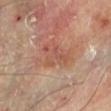Clinical impression:
Part of a total-body skin-imaging series; this lesion was reviewed on a skin check and was not flagged for biopsy.
Context:
The subject is a male about 70 years old. An algorithmic analysis of the crop reported a lesion color around L≈52 a*≈23 b*≈29 in CIELAB, a lesion–skin lightness drop of about 7, and a normalized border contrast of about 5. The software also gave a nevus-likeness score of about 0/100 and a lesion-detection confidence of about 100/100. Imaged with cross-polarized lighting. From the left lower leg. The lesion's longest dimension is about 4.5 mm. A roughly 15 mm field-of-view crop from a total-body skin photograph.